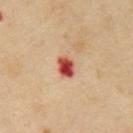follow-up: imaged on a skin check; not biopsied | subject: male, approximately 65 years of age | automated lesion analysis: an area of roughly 5 mm² and a shape eccentricity near 0.65; border irregularity of about 2 on a 0–10 scale and radial color variation of about 2.5; a lesion-detection confidence of about 100/100 | acquisition: ~15 mm crop, total-body skin-cancer survey | lighting: cross-polarized illumination.